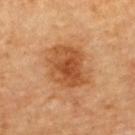Acquisition and patient details: Cropped from a whole-body photographic skin survey; the tile spans about 15 mm. Located on the upper back. The subject is a male aged approximately 85 or older. About 5 mm across.